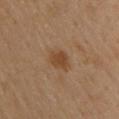Recorded during total-body skin imaging; not selected for excision or biopsy. A male patient, about 40 years old. The lesion is on the upper back. The lesion's longest dimension is about 3 mm. Cropped from a whole-body photographic skin survey; the tile spans about 15 mm.